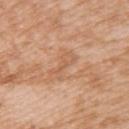Clinical impression:
The lesion was photographed on a routine skin check and not biopsied; there is no pathology result.
Clinical summary:
A 15 mm close-up tile from a total-body photography series done for melanoma screening. From the upper back. A female patient approximately 40 years of age. An algorithmic analysis of the crop reported a color-variation rating of about 1/10 and a peripheral color-asymmetry measure near 0.5. Measured at roughly 4 mm in maximum diameter.